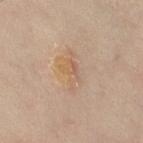Findings:
• image · ~15 mm tile from a whole-body skin photo
• size · ~5 mm (longest diameter)
• site · the right lower leg
• subject · female, about 55 years old
• tile lighting · cross-polarized
• image-analysis metrics · a lesion area of about 7 mm², an outline eccentricity of about 0.9 (0 = round, 1 = elongated), and two-axis asymmetry of about 0.45; border irregularity of about 5.5 on a 0–10 scale, internal color variation of about 6.5 on a 0–10 scale, and peripheral color asymmetry of about 2